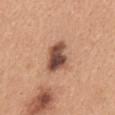A lesion tile, about 15 mm wide, cut from a 3D total-body photograph.
A female subject in their 30s.
An algorithmic analysis of the crop reported an area of roughly 9 mm², an outline eccentricity of about 0.75 (0 = round, 1 = elongated), and two-axis asymmetry of about 0.25. And it measured an average lesion color of about L≈49 a*≈22 b*≈28 (CIELAB), roughly 18 lightness units darker than nearby skin, and a lesion-to-skin contrast of about 12.5 (normalized; higher = more distinct). It also reported internal color variation of about 6.5 on a 0–10 scale and radial color variation of about 2. And it measured a nevus-likeness score of about 40/100 and lesion-presence confidence of about 100/100.
Located on the mid back.
Imaged with white-light lighting.
The recorded lesion diameter is about 4 mm.